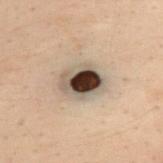Assessment: The lesion was tiled from a total-body skin photograph and was not biopsied. Acquisition and patient details: The lesion is located on the upper back. About 4.5 mm across. Cropped from a whole-body photographic skin survey; the tile spans about 15 mm. The tile uses cross-polarized illumination. Automated image analysis of the tile measured an area of roughly 12 mm² and a shape eccentricity near 0.7. And it measured a mean CIELAB color near L≈38 a*≈10 b*≈20, roughly 19 lightness units darker than nearby skin, and a lesion-to-skin contrast of about 15 (normalized; higher = more distinct). And it measured a border-irregularity rating of about 1.5/10 and peripheral color asymmetry of about 5. The subject is a male approximately 30 years of age.